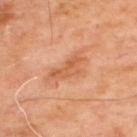{
  "biopsy_status": "not biopsied; imaged during a skin examination",
  "image": {
    "source": "total-body photography crop",
    "field_of_view_mm": 15
  },
  "site": "upper back",
  "patient": {
    "sex": "male",
    "age_approx": 65
  }
}